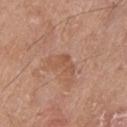* notes: total-body-photography surveillance lesion; no biopsy
* subject: male, in their 80s
* anatomic site: the leg
* size: about 3.5 mm
* tile lighting: white-light illumination
* imaging modality: ~15 mm crop, total-body skin-cancer survey